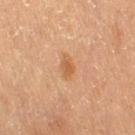Clinical impression: The lesion was photographed on a routine skin check and not biopsied; there is no pathology result. Context: The lesion is located on the right thigh. The total-body-photography lesion software estimated a lesion color around L≈51 a*≈20 b*≈34 in CIELAB, a lesion–skin lightness drop of about 7, and a lesion-to-skin contrast of about 6 (normalized; higher = more distinct). It also reported a classifier nevus-likeness of about 15/100 and a detector confidence of about 100 out of 100 that the crop contains a lesion. Approximately 2.5 mm at its widest. A 15 mm crop from a total-body photograph taken for skin-cancer surveillance. The patient is a female aged 53 to 57.